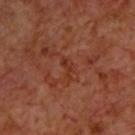Part of a total-body skin-imaging series; this lesion was reviewed on a skin check and was not flagged for biopsy.
Approximately 2.5 mm at its widest.
On the upper back.
A male subject aged approximately 70.
Cropped from a total-body skin-imaging series; the visible field is about 15 mm.
Captured under cross-polarized illumination.